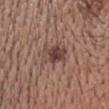Q: What is the imaging modality?
A: ~15 mm crop, total-body skin-cancer survey
Q: Patient demographics?
A: male, aged 38–42
Q: Where on the body is the lesion?
A: the head or neck